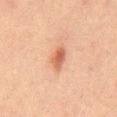Assessment:
Captured during whole-body skin photography for melanoma surveillance; the lesion was not biopsied.
Clinical summary:
Longest diameter approximately 3 mm. A 15 mm close-up extracted from a 3D total-body photography capture. The lesion is on the mid back. Automated tile analysis of the lesion measured a mean CIELAB color near L≈49 a*≈22 b*≈29, a lesion–skin lightness drop of about 10, and a normalized border contrast of about 7.5. It also reported border irregularity of about 2.5 on a 0–10 scale and internal color variation of about 3 on a 0–10 scale. The subject is a male aged approximately 65. This is a cross-polarized tile.